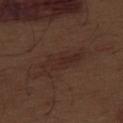  biopsy_status: not biopsied; imaged during a skin examination
  lesion_size:
    long_diameter_mm_approx: 6.5
  site: abdomen
  lighting: white-light
  image:
    source: total-body photography crop
    field_of_view_mm: 15
  patient:
    sex: male
    age_approx: 70